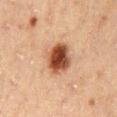Part of a total-body skin-imaging series; this lesion was reviewed on a skin check and was not flagged for biopsy. The lesion is on the lower back. The subject is a male about 50 years old. About 4 mm across. This image is a 15 mm lesion crop taken from a total-body photograph. Imaged with cross-polarized lighting. Automated image analysis of the tile measured a footprint of about 11 mm² and a shape eccentricity near 0.5. It also reported a classifier nevus-likeness of about 100/100.